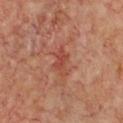Q: Was a biopsy performed?
A: no biopsy performed (imaged during a skin exam)
Q: Lesion size?
A: ~3.5 mm (longest diameter)
Q: Illumination type?
A: cross-polarized illumination
Q: Automated lesion metrics?
A: a lesion area of about 4.5 mm², an eccentricity of roughly 0.9, and a symmetry-axis asymmetry near 0.5; a mean CIELAB color near L≈45 a*≈29 b*≈29 and roughly 8 lightness units darker than nearby skin; a border-irregularity index near 5.5/10 and radial color variation of about 0; a classifier nevus-likeness of about 5/100
Q: Lesion location?
A: the chest
Q: What are the patient's age and sex?
A: female, about 45 years old
Q: How was this image acquired?
A: 15 mm crop, total-body photography